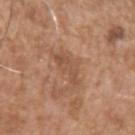<case>
  <biopsy_status>not biopsied; imaged during a skin examination</biopsy_status>
  <site>left upper arm</site>
  <lesion_size>
    <long_diameter_mm_approx>3.5</long_diameter_mm_approx>
  </lesion_size>
  <patient>
    <sex>male</sex>
    <age_approx>75</age_approx>
  </patient>
  <automated_metrics>
    <cielab_L>51</cielab_L>
    <cielab_a>21</cielab_a>
    <cielab_b>31</cielab_b>
    <vs_skin_darker_L>7.0</vs_skin_darker_L>
    <vs_skin_contrast_norm>5.5</vs_skin_contrast_norm>
    <border_irregularity_0_10>5.5</border_irregularity_0_10>
    <peripheral_color_asymmetry>0.5</peripheral_color_asymmetry>
    <nevus_likeness_0_100>0</nevus_likeness_0_100>
    <lesion_detection_confidence_0_100>100</lesion_detection_confidence_0_100>
  </automated_metrics>
  <image>
    <source>total-body photography crop</source>
    <field_of_view_mm>15</field_of_view_mm>
  </image>
  <lighting>white-light</lighting>
</case>